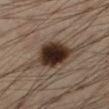  biopsy_status: not biopsied; imaged during a skin examination
  lighting: cross-polarized
  automated_metrics:
    area_mm2_approx: 14.0
    eccentricity: 0.65
    shape_asymmetry: 0.2
    vs_skin_darker_L: 18.0
  image:
    source: total-body photography crop
    field_of_view_mm: 15
  site: left lower leg
  lesion_size:
    long_diameter_mm_approx: 5.0
  patient:
    sex: male
    age_approx: 35